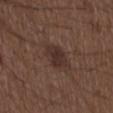Q: Was this lesion biopsied?
A: imaged on a skin check; not biopsied
Q: What kind of image is this?
A: 15 mm crop, total-body photography
Q: What is the anatomic site?
A: the chest
Q: Patient demographics?
A: male, aged 48 to 52